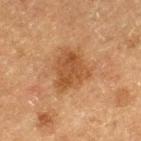Imaged during a routine full-body skin examination; the lesion was not biopsied and no histopathology is available.
Located on the left thigh.
This image is a 15 mm lesion crop taken from a total-body photograph.
Captured under cross-polarized illumination.
The recorded lesion diameter is about 4.5 mm.
A male patient, aged 73 to 77.